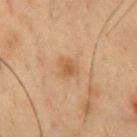• acquisition · 15 mm crop, total-body photography
• illumination · cross-polarized
• anatomic site · the chest
• subject · male, approximately 55 years of age